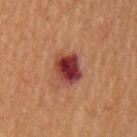Captured during whole-body skin photography for melanoma surveillance; the lesion was not biopsied.
The tile uses cross-polarized illumination.
A 15 mm close-up extracted from a 3D total-body photography capture.
On the right upper arm.
The lesion-visualizer software estimated an area of roughly 9 mm², a shape eccentricity near 0.55, and a symmetry-axis asymmetry near 0.15. It also reported an average lesion color of about L≈35 a*≈29 b*≈24 (CIELAB), a lesion–skin lightness drop of about 16, and a normalized lesion–skin contrast near 13.5. The analysis additionally found a border-irregularity rating of about 1.5/10, a color-variation rating of about 7/10, and radial color variation of about 2. The software also gave a nevus-likeness score of about 0/100 and lesion-presence confidence of about 100/100.
The subject is a male in their mid- to late 60s.
The recorded lesion diameter is about 3.5 mm.